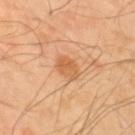The lesion is located on the upper back. A male subject about 40 years old. An algorithmic analysis of the crop reported an average lesion color of about L≈62 a*≈24 b*≈41 (CIELAB), a lesion–skin lightness drop of about 9, and a normalized border contrast of about 6. A lesion tile, about 15 mm wide, cut from a 3D total-body photograph. Approximately 3 mm at its widest. The tile uses cross-polarized illumination.